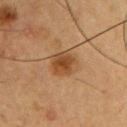Clinical impression:
This lesion was catalogued during total-body skin photography and was not selected for biopsy.
Background:
This is a cross-polarized tile. A roughly 15 mm field-of-view crop from a total-body skin photograph. A male subject, in their mid- to late 50s. The lesion's longest dimension is about 3 mm. The lesion is on the right upper arm. Automated image analysis of the tile measured a nevus-likeness score of about 95/100 and lesion-presence confidence of about 100/100.